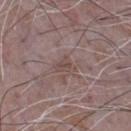{
  "biopsy_status": "not biopsied; imaged during a skin examination",
  "patient": {
    "sex": "male",
    "age_approx": 65
  },
  "automated_metrics": {
    "eccentricity": 0.65,
    "shape_asymmetry": 0.4,
    "cielab_L": 47,
    "cielab_a": 16,
    "cielab_b": 19,
    "vs_skin_darker_L": 6.0,
    "peripheral_color_asymmetry": 0.5,
    "nevus_likeness_0_100": 0,
    "lesion_detection_confidence_0_100": 90
  },
  "site": "chest",
  "image": {
    "source": "total-body photography crop",
    "field_of_view_mm": 15
  },
  "lighting": "white-light",
  "lesion_size": {
    "long_diameter_mm_approx": 2.5
  }
}